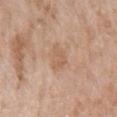lesion diameter = about 2.5 mm | image source = total-body-photography crop, ~15 mm field of view | illumination = white-light illumination | subject = female, aged around 70 | anatomic site = the left forearm.